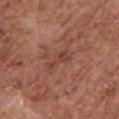Part of a total-body skin-imaging series; this lesion was reviewed on a skin check and was not flagged for biopsy. About 3.5 mm across. The lesion is on the chest. The patient is a male aged approximately 75. The lesion-visualizer software estimated a lesion color around L≈42 a*≈24 b*≈27 in CIELAB and a lesion-to-skin contrast of about 5.5 (normalized; higher = more distinct). The analysis additionally found a border-irregularity index near 6/10, internal color variation of about 1.5 on a 0–10 scale, and peripheral color asymmetry of about 0.5. This is a white-light tile. A roughly 15 mm field-of-view crop from a total-body skin photograph.